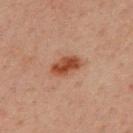Case summary:
- biopsy status — catalogued during a skin exam; not biopsied
- imaging modality — ~15 mm crop, total-body skin-cancer survey
- subject — male, aged 28–32
- size — about 4 mm
- site — the upper back
- automated lesion analysis — an area of roughly 7 mm², an outline eccentricity of about 0.8 (0 = round, 1 = elongated), and a symmetry-axis asymmetry near 0.15; a lesion color around L≈39 a*≈22 b*≈29 in CIELAB, roughly 10 lightness units darker than nearby skin, and a lesion-to-skin contrast of about 9.5 (normalized; higher = more distinct); a color-variation rating of about 4/10 and radial color variation of about 1.5; a classifier nevus-likeness of about 95/100